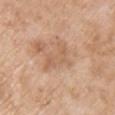biopsy status = catalogued during a skin exam; not biopsied
patient = female, aged 73–77
size = ~4.5 mm (longest diameter)
location = the right upper arm
imaging modality = 15 mm crop, total-body photography